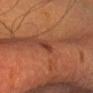<case>
  <biopsy_status>not biopsied; imaged during a skin examination</biopsy_status>
  <patient>
    <sex>female</sex>
    <age_approx>30</age_approx>
  </patient>
  <image>
    <source>total-body photography crop</source>
    <field_of_view_mm>15</field_of_view_mm>
  </image>
  <automated_metrics>
    <eccentricity>0.55</eccentricity>
    <shape_asymmetry>0.3</shape_asymmetry>
    <nevus_likeness_0_100>35</nevus_likeness_0_100>
    <lesion_detection_confidence_0_100>95</lesion_detection_confidence_0_100>
  </automated_metrics>
  <site>head or neck</site>
  <lighting>cross-polarized</lighting>
</case>